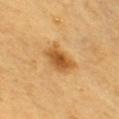Assessment:
Captured during whole-body skin photography for melanoma surveillance; the lesion was not biopsied.
Background:
The subject is a male aged approximately 60. Automated tile analysis of the lesion measured an area of roughly 9 mm², a shape eccentricity near 0.75, and a shape-asymmetry score of about 0.2 (0 = symmetric). This is a cross-polarized tile. A roughly 15 mm field-of-view crop from a total-body skin photograph. From the chest.